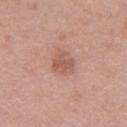<case>
  <biopsy_status>not biopsied; imaged during a skin examination</biopsy_status>
  <lesion_size>
    <long_diameter_mm_approx>3.0</long_diameter_mm_approx>
  </lesion_size>
  <site>chest</site>
  <image>
    <source>total-body photography crop</source>
    <field_of_view_mm>15</field_of_view_mm>
  </image>
  <patient>
    <sex>female</sex>
    <age_approx>40</age_approx>
  </patient>
  <automated_metrics>
    <area_mm2_approx>6.0</area_mm2_approx>
    <eccentricity>0.5</eccentricity>
    <lesion_detection_confidence_0_100>100</lesion_detection_confidence_0_100>
  </automated_metrics>
</case>